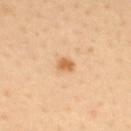| feature | finding |
|---|---|
| biopsy status | imaged on a skin check; not biopsied |
| automated lesion analysis | a footprint of about 2.5 mm², an outline eccentricity of about 0.7 (0 = round, 1 = elongated), and a shape-asymmetry score of about 0.2 (0 = symmetric); a lesion color around L≈64 a*≈24 b*≈42 in CIELAB, a lesion–skin lightness drop of about 12, and a lesion-to-skin contrast of about 8 (normalized; higher = more distinct) |
| size | ≈2 mm |
| lighting | cross-polarized illumination |
| acquisition | total-body-photography crop, ~15 mm field of view |
| location | the upper back |
| patient | male, aged around 30 |